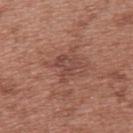Clinical summary:
A female subject aged approximately 40. On the upper back. A 15 mm close-up tile from a total-body photography series done for melanoma screening. Approximately 3 mm at its widest. The tile uses white-light illumination.